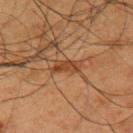Impression: The lesion was tiled from a total-body skin photograph and was not biopsied. Background: On the upper back. This is a cross-polarized tile. A region of skin cropped from a whole-body photographic capture, roughly 15 mm wide. The patient is a male aged approximately 60. The lesion's longest dimension is about 3 mm.